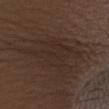Impression: The lesion was photographed on a routine skin check and not biopsied; there is no pathology result. Acquisition and patient details: Automated image analysis of the tile measured a peripheral color-asymmetry measure near 1. It also reported a nevus-likeness score of about 0/100 and a lesion-detection confidence of about 60/100. Captured under white-light illumination. The patient is a female aged 48–52. From the upper back. A roughly 15 mm field-of-view crop from a total-body skin photograph.